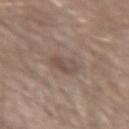Part of a total-body skin-imaging series; this lesion was reviewed on a skin check and was not flagged for biopsy.
The subject is a male about 30 years old.
The lesion is located on the left forearm.
A lesion tile, about 15 mm wide, cut from a 3D total-body photograph.